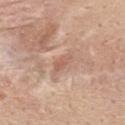{"biopsy_status": "not biopsied; imaged during a skin examination", "lesion_size": {"long_diameter_mm_approx": 2.5}, "site": "upper back", "patient": {"sex": "female", "age_approx": 35}, "image": {"source": "total-body photography crop", "field_of_view_mm": 15}, "lighting": "white-light"}